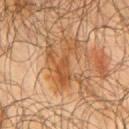Recorded during total-body skin imaging; not selected for excision or biopsy. A roughly 15 mm field-of-view crop from a total-body skin photograph. Automated tile analysis of the lesion measured a classifier nevus-likeness of about 0/100 and a lesion-detection confidence of about 80/100. Located on the right upper arm. Captured under cross-polarized illumination. A male patient approximately 65 years of age. The lesion's longest dimension is about 6 mm.